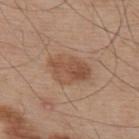Part of a total-body skin-imaging series; this lesion was reviewed on a skin check and was not flagged for biopsy.
About 4.5 mm across.
The total-body-photography lesion software estimated a lesion area of about 12 mm², an outline eccentricity of about 0.75 (0 = round, 1 = elongated), and two-axis asymmetry of about 0.2. And it measured roughly 9 lightness units darker than nearby skin and a normalized border contrast of about 7. The analysis additionally found an automated nevus-likeness rating near 65 out of 100 and lesion-presence confidence of about 100/100.
A male subject, in their mid- to late 50s.
On the upper back.
A region of skin cropped from a whole-body photographic capture, roughly 15 mm wide.